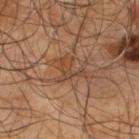No biopsy was performed on this lesion — it was imaged during a full skin examination and was not determined to be concerning.
The lesion's longest dimension is about 3.5 mm.
This is a cross-polarized tile.
A male patient about 65 years old.
A 15 mm close-up tile from a total-body photography series done for melanoma screening.
The lesion is on the upper back.